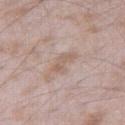follow-up — no biopsy performed (imaged during a skin exam); acquisition — ~15 mm tile from a whole-body skin photo; lesion diameter — ≈3.5 mm; location — the right thigh; subject — male, in their mid- to late 40s.